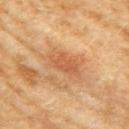workup = catalogued during a skin exam; not biopsied | lesion diameter = ≈4 mm | location = the right upper arm | automated metrics = a classifier nevus-likeness of about 20/100 and a lesion-detection confidence of about 100/100 | patient = female, aged around 60 | imaging modality = 15 mm crop, total-body photography.